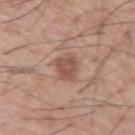| feature | finding |
|---|---|
| follow-up | no biopsy performed (imaged during a skin exam) |
| lighting | white-light illumination |
| acquisition | total-body-photography crop, ~15 mm field of view |
| patient | male, aged around 55 |
| location | the left upper arm |
| diameter | about 3.5 mm |